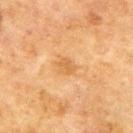anatomic site=the upper back
image source=15 mm crop, total-body photography
size=≈3 mm
subject=male, approximately 70 years of age